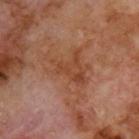notes: imaged on a skin check; not biopsied | body site: the back | imaging modality: total-body-photography crop, ~15 mm field of view | patient: male, aged 68 to 72 | size: about 7 mm | illumination: cross-polarized | image-analysis metrics: a footprint of about 20 mm², an outline eccentricity of about 0.6 (0 = round, 1 = elongated), and a shape-asymmetry score of about 0.6 (0 = symmetric).